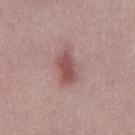| key | value |
|---|---|
| notes | catalogued during a skin exam; not biopsied |
| illumination | white-light |
| TBP lesion metrics | a symmetry-axis asymmetry near 0.25; an average lesion color of about L≈52 a*≈23 b*≈21 (CIELAB), a lesion–skin lightness drop of about 11, and a lesion-to-skin contrast of about 7.5 (normalized; higher = more distinct); border irregularity of about 3 on a 0–10 scale, a within-lesion color-variation index near 4/10, and peripheral color asymmetry of about 1; an automated nevus-likeness rating near 80 out of 100 |
| patient | male, in their 30s |
| size | ≈4.5 mm |
| imaging modality | total-body-photography crop, ~15 mm field of view |